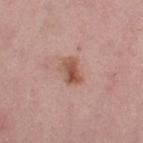Assessment:
The lesion was tiled from a total-body skin photograph and was not biopsied.
Image and clinical context:
From the right thigh. A female patient aged around 50. A 15 mm close-up tile from a total-body photography series done for melanoma screening.